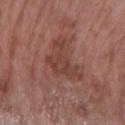follow-up: no biopsy performed (imaged during a skin exam) | tile lighting: white-light illumination | site: the left forearm | imaging modality: 15 mm crop, total-body photography | patient: female, aged around 60 | automated metrics: an area of roughly 9.5 mm², an outline eccentricity of about 0.75 (0 = round, 1 = elongated), and a symmetry-axis asymmetry near 0.7; a mean CIELAB color near L≈41 a*≈23 b*≈25, a lesion–skin lightness drop of about 8, and a lesion-to-skin contrast of about 6.5 (normalized; higher = more distinct); a border-irregularity index near 8.5/10, internal color variation of about 2.5 on a 0–10 scale, and peripheral color asymmetry of about 1.